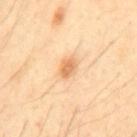follow-up = catalogued during a skin exam; not biopsied | patient = male, aged around 50 | site = the back | automated metrics = a mean CIELAB color near L≈71 a*≈22 b*≈42 and a normalized lesion–skin contrast near 7; a color-variation rating of about 2/10 and a peripheral color-asymmetry measure near 1; lesion-presence confidence of about 100/100 | lighting = cross-polarized illumination | image source = ~15 mm crop, total-body skin-cancer survey.